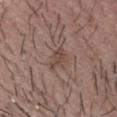Captured during whole-body skin photography for melanoma surveillance; the lesion was not biopsied. The total-body-photography lesion software estimated a lesion–skin lightness drop of about 8 and a normalized border contrast of about 6.5. The software also gave border irregularity of about 3.5 on a 0–10 scale and peripheral color asymmetry of about 0.5. About 3 mm across. The patient is a male aged 28 to 32. A 15 mm close-up extracted from a 3D total-body photography capture.